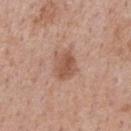{"biopsy_status": "not biopsied; imaged during a skin examination", "lighting": "white-light", "image": {"source": "total-body photography crop", "field_of_view_mm": 15}, "site": "right forearm", "patient": {"sex": "female", "age_approx": 40}, "lesion_size": {"long_diameter_mm_approx": 3.5}}